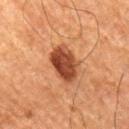The lesion was photographed on a routine skin check and not biopsied; there is no pathology result.
Imaged with cross-polarized lighting.
Measured at roughly 4.5 mm in maximum diameter.
A 15 mm close-up extracted from a 3D total-body photography capture.
A male subject, aged around 80.
On the leg.
Automated image analysis of the tile measured a footprint of about 11 mm², an eccentricity of roughly 0.7, and two-axis asymmetry of about 0.15. It also reported a nevus-likeness score of about 95/100.